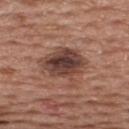workup: imaged on a skin check; not biopsied
image: ~15 mm tile from a whole-body skin photo
lighting: white-light illumination
patient: male, approximately 55 years of age
lesion size: ~5 mm (longest diameter)
site: the upper back